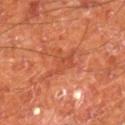The lesion was photographed on a routine skin check and not biopsied; there is no pathology result. A male subject, aged approximately 65. From the right lower leg. This image is a 15 mm lesion crop taken from a total-body photograph. The lesion's longest dimension is about 4.5 mm. An algorithmic analysis of the crop reported an area of roughly 7 mm², a shape eccentricity near 0.7, and two-axis asymmetry of about 0.45. And it measured a border-irregularity rating of about 6.5/10, a color-variation rating of about 2/10, and peripheral color asymmetry of about 0.5. The software also gave a classifier nevus-likeness of about 0/100 and lesion-presence confidence of about 100/100. Imaged with cross-polarized lighting.